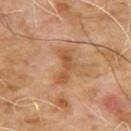Assessment:
Recorded during total-body skin imaging; not selected for excision or biopsy.
Context:
A 15 mm close-up tile from a total-body photography series done for melanoma screening. The subject is a male aged around 65. An algorithmic analysis of the crop reported a border-irregularity index near 5.5/10, internal color variation of about 2 on a 0–10 scale, and a peripheral color-asymmetry measure near 0.5. The lesion's longest dimension is about 4 mm. Located on the upper back.